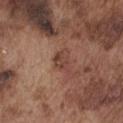{
  "biopsy_status": "not biopsied; imaged during a skin examination",
  "image": {
    "source": "total-body photography crop",
    "field_of_view_mm": 15
  },
  "automated_metrics": {
    "area_mm2_approx": 3.5,
    "eccentricity": 0.8,
    "cielab_L": 42,
    "cielab_a": 22,
    "cielab_b": 26,
    "vs_skin_darker_L": 9.0,
    "color_variation_0_10": 2.0,
    "peripheral_color_asymmetry": 0.5,
    "nevus_likeness_0_100": 5,
    "lesion_detection_confidence_0_100": 100
  },
  "lesion_size": {
    "long_diameter_mm_approx": 3.0
  },
  "lighting": "white-light",
  "site": "chest",
  "patient": {
    "sex": "male",
    "age_approx": 75
  }
}